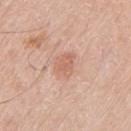Q: Was a biopsy performed?
A: no biopsy performed (imaged during a skin exam)
Q: Where on the body is the lesion?
A: the right upper arm
Q: What did automated image analysis measure?
A: a lesion color around L≈63 a*≈23 b*≈30 in CIELAB, a lesion–skin lightness drop of about 8, and a lesion-to-skin contrast of about 5.5 (normalized; higher = more distinct); a border-irregularity rating of about 2.5/10, a color-variation rating of about 1.5/10, and radial color variation of about 0.5; a classifier nevus-likeness of about 25/100 and lesion-presence confidence of about 100/100
Q: Patient demographics?
A: male, aged 78–82
Q: Lesion size?
A: about 3 mm
Q: How was this image acquired?
A: ~15 mm tile from a whole-body skin photo
Q: Illumination type?
A: white-light